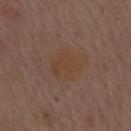A male subject aged approximately 70. A 15 mm close-up tile from a total-body photography series done for melanoma screening. From the mid back.